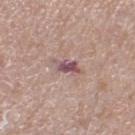biopsy status: total-body-photography surveillance lesion; no biopsy
anatomic site: the leg
image: 15 mm crop, total-body photography
subject: male, in their mid-70s
lighting: white-light illumination
lesion size: ≈4 mm